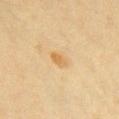<tbp_lesion>
  <biopsy_status>not biopsied; imaged during a skin examination</biopsy_status>
  <image>
    <source>total-body photography crop</source>
    <field_of_view_mm>15</field_of_view_mm>
  </image>
  <patient>
    <sex>female</sex>
    <age_approx>30</age_approx>
  </patient>
  <lesion_size>
    <long_diameter_mm_approx>2.5</long_diameter_mm_approx>
  </lesion_size>
  <site>chest</site>
  <lighting>cross-polarized</lighting>
</tbp_lesion>